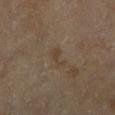workup = no biopsy performed (imaged during a skin exam) | diameter = about 3 mm | image source = total-body-photography crop, ~15 mm field of view | anatomic site = the right lower leg | illumination = cross-polarized illumination | automated metrics = a lesion area of about 3 mm² and an eccentricity of roughly 0.9; a lesion–skin lightness drop of about 5 and a normalized lesion–skin contrast near 5.5; a classifier nevus-likeness of about 0/100 | patient = male, in their mid-60s.